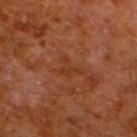Imaged during a routine full-body skin examination; the lesion was not biopsied and no histopathology is available.
The lesion is located on the left lower leg.
A lesion tile, about 15 mm wide, cut from a 3D total-body photograph.
The patient is a male aged 78–82.